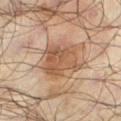Recorded during total-body skin imaging; not selected for excision or biopsy.
Cropped from a total-body skin-imaging series; the visible field is about 15 mm.
The tile uses cross-polarized illumination.
About 5 mm across.
The lesion-visualizer software estimated a lesion area of about 16 mm², an outline eccentricity of about 0.4 (0 = round, 1 = elongated), and two-axis asymmetry of about 0.25. The analysis additionally found a lesion color around L≈51 a*≈19 b*≈31 in CIELAB, about 10 CIELAB-L* units darker than the surrounding skin, and a normalized lesion–skin contrast near 7.5.
The subject is a male roughly 65 years of age.
Located on the leg.